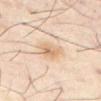Assessment:
No biopsy was performed on this lesion — it was imaged during a full skin examination and was not determined to be concerning.
Background:
From the abdomen. The lesion-visualizer software estimated an area of roughly 6 mm² and an outline eccentricity of about 0.7 (0 = round, 1 = elongated). It also reported an average lesion color of about L≈71 a*≈17 b*≈35 (CIELAB). The software also gave a border-irregularity index near 2.5/10, a color-variation rating of about 6/10, and radial color variation of about 2. It also reported an automated nevus-likeness rating near 50 out of 100. A male subject in their mid- to late 50s. Longest diameter approximately 3.5 mm. Captured under cross-polarized illumination. Cropped from a whole-body photographic skin survey; the tile spans about 15 mm.